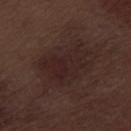| feature | finding |
|---|---|
| biopsy status | no biopsy performed (imaged during a skin exam) |
| body site | the left thigh |
| tile lighting | white-light illumination |
| patient | male, aged 68–72 |
| image source | 15 mm crop, total-body photography |